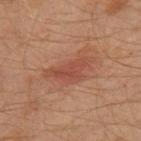Q: Was a biopsy performed?
A: total-body-photography surveillance lesion; no biopsy
Q: Patient demographics?
A: male, in their 30s
Q: What is the imaging modality?
A: ~15 mm crop, total-body skin-cancer survey
Q: What is the anatomic site?
A: the left leg
Q: Automated lesion metrics?
A: a lesion area of about 9.5 mm², a shape eccentricity near 0.9, and a symmetry-axis asymmetry near 0.5
Q: What is the lesion's diameter?
A: about 5.5 mm
Q: Illumination type?
A: cross-polarized illumination